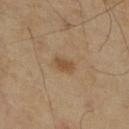Captured during whole-body skin photography for melanoma surveillance; the lesion was not biopsied.
This image is a 15 mm lesion crop taken from a total-body photograph.
The subject is a male in their 70s.
The lesion is on the right lower leg.